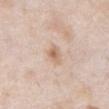This lesion was catalogued during total-body skin photography and was not selected for biopsy. This is a white-light tile. A male subject aged 53 to 57. A lesion tile, about 15 mm wide, cut from a 3D total-body photograph. The recorded lesion diameter is about 2.5 mm. The lesion is located on the chest.